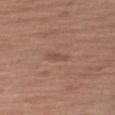body site — the arm; tile lighting — white-light illumination; diameter — ≈2.5 mm; acquisition — ~15 mm tile from a whole-body skin photo; patient — male, about 60 years old.